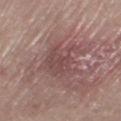Impression:
The lesion was photographed on a routine skin check and not biopsied; there is no pathology result.